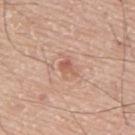biopsy status: no biopsy performed (imaged during a skin exam)
body site: the upper back
patient: male, in their mid- to late 70s
lighting: white-light
size: ≈2.5 mm
image source: 15 mm crop, total-body photography